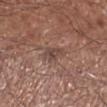Clinical impression: Captured during whole-body skin photography for melanoma surveillance; the lesion was not biopsied. Context: The subject is a male aged 68–72. The total-body-photography lesion software estimated an average lesion color of about L≈44 a*≈18 b*≈23 (CIELAB), about 8 CIELAB-L* units darker than the surrounding skin, and a lesion-to-skin contrast of about 6.5 (normalized; higher = more distinct). This image is a 15 mm lesion crop taken from a total-body photograph. The lesion is on the right lower leg. The recorded lesion diameter is about 2.5 mm. Captured under white-light illumination.